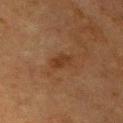Assessment:
Part of a total-body skin-imaging series; this lesion was reviewed on a skin check and was not flagged for biopsy.
Background:
Located on the chest. The patient is a male aged 73 to 77. A roughly 15 mm field-of-view crop from a total-body skin photograph. The lesion's longest dimension is about 3 mm.